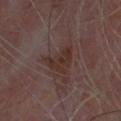<case>
  <biopsy_status>not biopsied; imaged during a skin examination</biopsy_status>
  <image>
    <source>total-body photography crop</source>
    <field_of_view_mm>15</field_of_view_mm>
  </image>
  <automated_metrics>
    <nevus_likeness_0_100>0</nevus_likeness_0_100>
    <lesion_detection_confidence_0_100>100</lesion_detection_confidence_0_100>
  </automated_metrics>
  <patient>
    <sex>male</sex>
    <age_approx>60</age_approx>
  </patient>
  <lighting>cross-polarized</lighting>
  <site>head or neck</site>
</case>